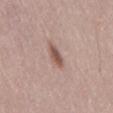| feature | finding |
|---|---|
| workup | imaged on a skin check; not biopsied |
| subject | female, in their 40s |
| acquisition | total-body-photography crop, ~15 mm field of view |
| lighting | white-light |
| body site | the lower back |
| image-analysis metrics | an outline eccentricity of about 0.85 (0 = round, 1 = elongated) and a shape-asymmetry score of about 0.2 (0 = symmetric); a mean CIELAB color near L≈53 a*≈19 b*≈24, about 12 CIELAB-L* units darker than the surrounding skin, and a normalized lesion–skin contrast near 8.5 |